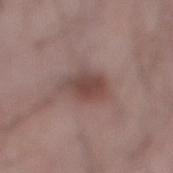Clinical impression: Imaged during a routine full-body skin examination; the lesion was not biopsied and no histopathology is available. Image and clinical context: Cropped from a whole-body photographic skin survey; the tile spans about 15 mm. Captured under white-light illumination. On the right lower leg. A male patient, roughly 25 years of age. Longest diameter approximately 5.5 mm.